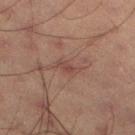Q: What kind of image is this?
A: total-body-photography crop, ~15 mm field of view
Q: Who is the patient?
A: male, in their 60s
Q: How was the tile lit?
A: cross-polarized
Q: What is the lesion's diameter?
A: ≈2.5 mm
Q: What did automated image analysis measure?
A: a footprint of about 2.5 mm², an eccentricity of roughly 0.85, and two-axis asymmetry of about 0.3; a mean CIELAB color near L≈39 a*≈19 b*≈21, roughly 6 lightness units darker than nearby skin, and a lesion-to-skin contrast of about 5.5 (normalized; higher = more distinct); border irregularity of about 3 on a 0–10 scale